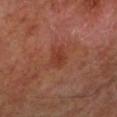Findings:
- biopsy status — no biopsy performed (imaged during a skin exam)
- patient — male, aged approximately 65
- anatomic site — the leg
- imaging modality — ~15 mm crop, total-body skin-cancer survey
- illumination — cross-polarized illumination
- lesion size — ~2.5 mm (longest diameter)